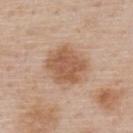follow-up: total-body-photography surveillance lesion; no biopsy | illumination: white-light illumination | body site: the upper back | patient: male, aged 58 to 62 | image source: 15 mm crop, total-body photography | lesion size: ~5 mm (longest diameter).